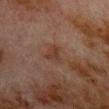| feature | finding |
|---|---|
| workup | no biopsy performed (imaged during a skin exam) |
| site | the upper back |
| imaging modality | total-body-photography crop, ~15 mm field of view |
| subject | male, roughly 80 years of age |